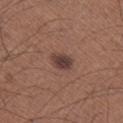Q: Is there a histopathology result?
A: no biopsy performed (imaged during a skin exam)
Q: Lesion location?
A: the right lower leg
Q: Patient demographics?
A: male, aged 63 to 67
Q: What is the imaging modality?
A: ~15 mm crop, total-body skin-cancer survey
Q: Illumination type?
A: white-light illumination
Q: What did automated image analysis measure?
A: a lesion area of about 5 mm², a shape eccentricity near 0.75, and a shape-asymmetry score of about 0.2 (0 = symmetric); lesion-presence confidence of about 100/100
Q: Lesion size?
A: ~3 mm (longest diameter)